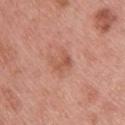patient=male, aged approximately 60; body site=the upper back; image=~15 mm tile from a whole-body skin photo; lighting=white-light illumination; size=about 2.5 mm.